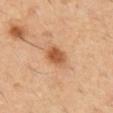Q: Is there a histopathology result?
A: catalogued during a skin exam; not biopsied
Q: What is the imaging modality?
A: ~15 mm tile from a whole-body skin photo
Q: What is the anatomic site?
A: the left thigh
Q: Who is the patient?
A: male, aged around 60
Q: Automated lesion metrics?
A: a lesion area of about 5.5 mm², an eccentricity of roughly 0.6, and two-axis asymmetry of about 0.15; a lesion-detection confidence of about 100/100
Q: Lesion size?
A: ~3 mm (longest diameter)